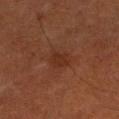Impression: The lesion was photographed on a routine skin check and not biopsied; there is no pathology result. Clinical summary: On the right forearm. A close-up tile cropped from a whole-body skin photograph, about 15 mm across. A male subject approximately 75 years of age.